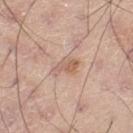{
  "biopsy_status": "not biopsied; imaged during a skin examination",
  "automated_metrics": {
    "area_mm2_approx": 5.0,
    "eccentricity": 0.8,
    "shape_asymmetry": 0.3,
    "cielab_L": 61,
    "cielab_a": 19,
    "cielab_b": 28,
    "vs_skin_darker_L": 9.0,
    "vs_skin_contrast_norm": 6.0,
    "border_irregularity_0_10": 3.5,
    "color_variation_0_10": 3.0,
    "peripheral_color_asymmetry": 1.0,
    "nevus_likeness_0_100": 5,
    "lesion_detection_confidence_0_100": 100
  },
  "lesion_size": {
    "long_diameter_mm_approx": 3.5
  },
  "lighting": "white-light",
  "image": {
    "source": "total-body photography crop",
    "field_of_view_mm": 15
  },
  "patient": {
    "sex": "male",
    "age_approx": 45
  },
  "site": "left thigh"
}